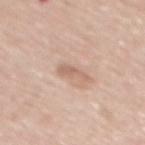Clinical impression:
Part of a total-body skin-imaging series; this lesion was reviewed on a skin check and was not flagged for biopsy.
Clinical summary:
The tile uses white-light illumination. A 15 mm close-up extracted from a 3D total-body photography capture. The lesion is located on the mid back. A male patient aged around 60. Automated image analysis of the tile measured an eccentricity of roughly 0.9 and two-axis asymmetry of about 0.45. The software also gave a lesion–skin lightness drop of about 9 and a normalized border contrast of about 5.5. And it measured a border-irregularity index near 5/10, internal color variation of about 0.5 on a 0–10 scale, and a peripheral color-asymmetry measure near 0. And it measured a nevus-likeness score of about 0/100 and a lesion-detection confidence of about 100/100. The lesion's longest dimension is about 3 mm.